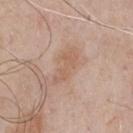A male patient in their mid- to late 60s.
From the chest.
About 4.5 mm across.
A region of skin cropped from a whole-body photographic capture, roughly 15 mm wide.